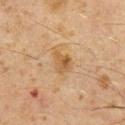Assessment: No biopsy was performed on this lesion — it was imaged during a full skin examination and was not determined to be concerning. Background: Measured at roughly 2.5 mm in maximum diameter. A male subject aged 58 to 62. The tile uses cross-polarized illumination. A region of skin cropped from a whole-body photographic capture, roughly 15 mm wide. Located on the chest.